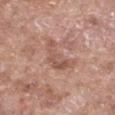Q: Is there a histopathology result?
A: no biopsy performed (imaged during a skin exam)
Q: What kind of image is this?
A: ~15 mm tile from a whole-body skin photo
Q: Patient demographics?
A: female, aged around 75
Q: Lesion size?
A: ≈5 mm
Q: Lesion location?
A: the front of the torso